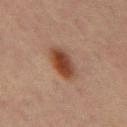Case summary:
- biopsy status — catalogued during a skin exam; not biopsied
- acquisition — total-body-photography crop, ~15 mm field of view
- body site — the abdomen
- illumination — cross-polarized illumination
- automated metrics — an area of roughly 9 mm², a shape eccentricity near 0.8, and a shape-asymmetry score of about 0.15 (0 = symmetric); a classifier nevus-likeness of about 100/100
- diameter — ~4 mm (longest diameter)
- patient — male, aged approximately 70